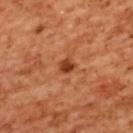notes: imaged on a skin check; not biopsied
subject: female, aged 53–57
diameter: about 2 mm
location: the upper back
lighting: cross-polarized
automated metrics: an average lesion color of about L≈43 a*≈32 b*≈39 (CIELAB), roughly 13 lightness units darker than nearby skin, and a lesion-to-skin contrast of about 9.5 (normalized; higher = more distinct); a border-irregularity rating of about 2/10, a within-lesion color-variation index near 2/10, and peripheral color asymmetry of about 1
image: ~15 mm tile from a whole-body skin photo